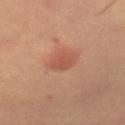Recorded during total-body skin imaging; not selected for excision or biopsy.
The lesion is located on the right upper arm.
A roughly 15 mm field-of-view crop from a total-body skin photograph.
Imaged with cross-polarized lighting.
A male patient aged 63 to 67.
Approximately 3 mm at its widest.